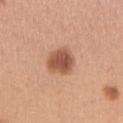The patient is a female approximately 30 years of age. Longest diameter approximately 4 mm. An algorithmic analysis of the crop reported a mean CIELAB color near L≈54 a*≈24 b*≈32, about 14 CIELAB-L* units darker than the surrounding skin, and a normalized lesion–skin contrast near 9.5. A 15 mm close-up extracted from a 3D total-body photography capture. The lesion is on the arm. This is a white-light tile.